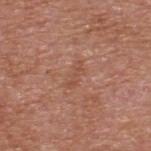follow-up = catalogued during a skin exam; not biopsied
subject = male, about 65 years old
size = about 3 mm
image-analysis metrics = a border-irregularity index near 5.5/10, a within-lesion color-variation index near 0/10, and radial color variation of about 0; a nevus-likeness score of about 0/100
acquisition = ~15 mm crop, total-body skin-cancer survey
lighting = white-light
location = the upper back